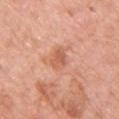<lesion>
<biopsy_status>not biopsied; imaged during a skin examination</biopsy_status>
<lesion_size>
  <long_diameter_mm_approx>2.5</long_diameter_mm_approx>
</lesion_size>
<lighting>white-light</lighting>
<image>
  <source>total-body photography crop</source>
  <field_of_view_mm>15</field_of_view_mm>
</image>
<site>chest</site>
<patient>
  <sex>male</sex>
  <age_approx>55</age_approx>
</patient>
</lesion>